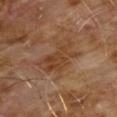image:
  source: total-body photography crop
  field_of_view_mm: 15
lighting: cross-polarized
patient:
  sex: male
  age_approx: 60
lesion_size:
  long_diameter_mm_approx: 6.0
site: chest
automated_metrics:
  area_mm2_approx: 11.0
  eccentricity: 0.85
  shape_asymmetry: 0.4
  cielab_L: 39
  cielab_a: 19
  cielab_b: 31
  vs_skin_darker_L: 7.0
  vs_skin_contrast_norm: 7.0
  nevus_likeness_0_100: 0
  lesion_detection_confidence_0_100: 100